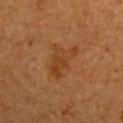image-analysis metrics: a classifier nevus-likeness of about 5/100 and a detector confidence of about 100 out of 100 that the crop contains a lesion
anatomic site: the left upper arm
imaging modality: 15 mm crop, total-body photography
tile lighting: cross-polarized illumination
size: ~4.5 mm (longest diameter)
subject: male, aged approximately 55